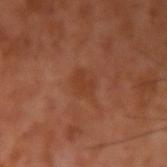follow-up: imaged on a skin check; not biopsied
illumination: cross-polarized illumination
TBP lesion metrics: a footprint of about 4 mm² and an eccentricity of roughly 0.75; a border-irregularity index near 2.5/10; an automated nevus-likeness rating near 0 out of 100 and a lesion-detection confidence of about 100/100
diameter: ~2.5 mm (longest diameter)
subject: male, about 55 years old
site: the arm
image source: ~15 mm tile from a whole-body skin photo